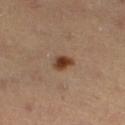{"biopsy_status": "not biopsied; imaged during a skin examination", "site": "left lower leg", "image": {"source": "total-body photography crop", "field_of_view_mm": 15}, "lesion_size": {"long_diameter_mm_approx": 2.5}, "lighting": "cross-polarized", "patient": {"sex": "female", "age_approx": 35}}